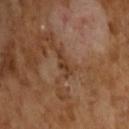Assessment: No biopsy was performed on this lesion — it was imaged during a full skin examination and was not determined to be concerning. Clinical summary: A 15 mm close-up tile from a total-body photography series done for melanoma screening. The recorded lesion diameter is about 3 mm. A male subject, aged approximately 65. This is a cross-polarized tile.